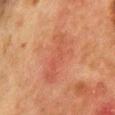No biopsy was performed on this lesion — it was imaged during a full skin examination and was not determined to be concerning.
The patient is a female approximately 50 years of age.
Measured at roughly 6.5 mm in maximum diameter.
A 15 mm close-up extracted from a 3D total-body photography capture.
The lesion is located on the front of the torso.